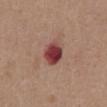A 15 mm close-up tile from a total-body photography series done for melanoma screening.
A male subject, aged 73–77.
The lesion is located on the chest.